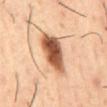{
  "biopsy_status": "not biopsied; imaged during a skin examination",
  "lesion_size": {
    "long_diameter_mm_approx": 6.0
  },
  "image": {
    "source": "total-body photography crop",
    "field_of_view_mm": 15
  },
  "site": "abdomen",
  "patient": {
    "sex": "male",
    "age_approx": 55
  },
  "automated_metrics": {
    "area_mm2_approx": 14.0,
    "eccentricity": 0.85,
    "shape_asymmetry": 0.2
  },
  "lighting": "cross-polarized"
}